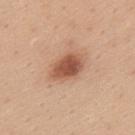- workup: imaged on a skin check; not biopsied
- lesion size: about 4.5 mm
- site: the upper back
- patient: male, in their mid- to late 30s
- image: 15 mm crop, total-body photography
- illumination: white-light